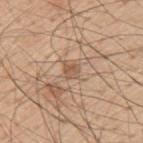Recorded during total-body skin imaging; not selected for excision or biopsy. Located on the arm. A 15 mm crop from a total-body photograph taken for skin-cancer surveillance. Longest diameter approximately 2 mm. An algorithmic analysis of the crop reported a shape-asymmetry score of about 0.3 (0 = symmetric). The analysis additionally found a detector confidence of about 70 out of 100 that the crop contains a lesion. A male patient, about 55 years old. Captured under white-light illumination.